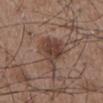Assessment: Recorded during total-body skin imaging; not selected for excision or biopsy. Image and clinical context: A close-up tile cropped from a whole-body skin photograph, about 15 mm across. On the abdomen. Measured at roughly 6.5 mm in maximum diameter. The total-body-photography lesion software estimated an average lesion color of about L≈42 a*≈17 b*≈24 (CIELAB). It also reported a nevus-likeness score of about 85/100 and a lesion-detection confidence of about 100/100. This is a white-light tile. A male patient, in their mid- to late 50s.